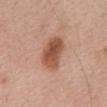Imaged during a routine full-body skin examination; the lesion was not biopsied and no histopathology is available. On the mid back. This is a white-light tile. Automated image analysis of the tile measured an area of roughly 11 mm², an outline eccentricity of about 0.8 (0 = round, 1 = elongated), and two-axis asymmetry of about 0.15. A roughly 15 mm field-of-view crop from a total-body skin photograph. A male patient, in their 70s.